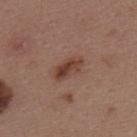{
  "patient": {
    "sex": "female",
    "age_approx": 45
  },
  "lighting": "white-light",
  "site": "back",
  "automated_metrics": {
    "eccentricity": 0.85,
    "shape_asymmetry": 0.2,
    "border_irregularity_0_10": 2.0,
    "color_variation_0_10": 6.0,
    "peripheral_color_asymmetry": 2.0,
    "lesion_detection_confidence_0_100": 100
  },
  "image": {
    "source": "total-body photography crop",
    "field_of_view_mm": 15
  },
  "lesion_size": {
    "long_diameter_mm_approx": 4.0
  }
}